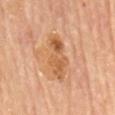Q: Was this lesion biopsied?
A: catalogued during a skin exam; not biopsied
Q: What kind of image is this?
A: total-body-photography crop, ~15 mm field of view
Q: What is the anatomic site?
A: the mid back
Q: What lighting was used for the tile?
A: cross-polarized
Q: Automated lesion metrics?
A: an average lesion color of about L≈57 a*≈22 b*≈38 (CIELAB), about 10 CIELAB-L* units darker than the surrounding skin, and a lesion-to-skin contrast of about 7.5 (normalized; higher = more distinct); a border-irregularity index near 4.5/10, a color-variation rating of about 6/10, and radial color variation of about 2; a classifier nevus-likeness of about 0/100 and lesion-presence confidence of about 100/100
Q: Patient demographics?
A: male, about 85 years old or older